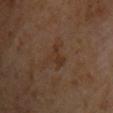Assessment: Captured during whole-body skin photography for melanoma surveillance; the lesion was not biopsied. Image and clinical context: The subject is a male approximately 60 years of age. Approximately 3 mm at its widest. A roughly 15 mm field-of-view crop from a total-body skin photograph. Located on the chest. An algorithmic analysis of the crop reported a symmetry-axis asymmetry near 0.55. This is a cross-polarized tile.